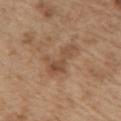Clinical summary:
From the arm. A close-up tile cropped from a whole-body skin photograph, about 15 mm across. The subject is a male about 70 years old. Measured at roughly 4.5 mm in maximum diameter.